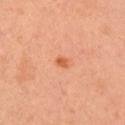<lesion>
  <patient>
    <sex>female</sex>
    <age_approx>30</age_approx>
  </patient>
  <site>right upper arm</site>
  <image>
    <source>total-body photography crop</source>
    <field_of_view_mm>15</field_of_view_mm>
  </image>
</lesion>